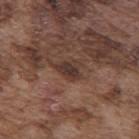Q: Is there a histopathology result?
A: total-body-photography surveillance lesion; no biopsy
Q: What is the anatomic site?
A: the mid back
Q: What are the patient's age and sex?
A: male, in their mid-70s
Q: What is the imaging modality?
A: total-body-photography crop, ~15 mm field of view
Q: Automated lesion metrics?
A: a border-irregularity index near 3.5/10, internal color variation of about 3.5 on a 0–10 scale, and radial color variation of about 1
Q: What lighting was used for the tile?
A: white-light